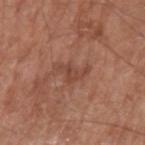Q: Was a biopsy performed?
A: total-body-photography surveillance lesion; no biopsy
Q: What kind of image is this?
A: total-body-photography crop, ~15 mm field of view
Q: Lesion location?
A: the left upper arm
Q: Who is the patient?
A: male, about 65 years old